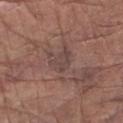* workup · total-body-photography surveillance lesion; no biopsy
* lesion size · ~3 mm (longest diameter)
* imaging modality · 15 mm crop, total-body photography
* tile lighting · white-light
* subject · male, aged approximately 80
* anatomic site · the right upper arm